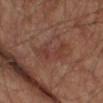Q: Was this lesion biopsied?
A: imaged on a skin check; not biopsied
Q: How large is the lesion?
A: about 5.5 mm
Q: What lighting was used for the tile?
A: cross-polarized
Q: What are the patient's age and sex?
A: male, aged approximately 65
Q: Where on the body is the lesion?
A: the left upper arm
Q: What is the imaging modality?
A: total-body-photography crop, ~15 mm field of view